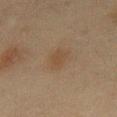About 3 mm across.
The patient is a male in their mid-40s.
A 15 mm crop from a total-body photograph taken for skin-cancer surveillance.
The lesion-visualizer software estimated an average lesion color of about L≈37 a*≈13 b*≈26 (CIELAB) and a lesion–skin lightness drop of about 5. The software also gave a classifier nevus-likeness of about 70/100 and lesion-presence confidence of about 100/100.
On the chest.